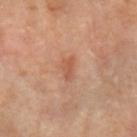{"lesion_size": {"long_diameter_mm_approx": 3.0}, "image": {"source": "total-body photography crop", "field_of_view_mm": 15}, "site": "left forearm", "patient": {"sex": "female", "age_approx": 40}}